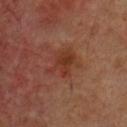image: ~15 mm crop, total-body skin-cancer survey
image-analysis metrics: a lesion area of about 7.5 mm², an outline eccentricity of about 0.7 (0 = round, 1 = elongated), and two-axis asymmetry of about 0.4; a lesion–skin lightness drop of about 7; border irregularity of about 5.5 on a 0–10 scale and a color-variation rating of about 3.5/10; an automated nevus-likeness rating near 0 out of 100 and a detector confidence of about 100 out of 100 that the crop contains a lesion
tile lighting: cross-polarized
size: ~4 mm (longest diameter)
anatomic site: the chest
patient: male, aged 48 to 52